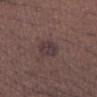notes = imaged on a skin check; not biopsied | acquisition = ~15 mm tile from a whole-body skin photo | subject = male, roughly 40 years of age | lesion diameter = ≈3 mm | location = the left forearm.